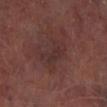The lesion was photographed on a routine skin check and not biopsied; there is no pathology result. The recorded lesion diameter is about 4.5 mm. The lesion-visualizer software estimated an automated nevus-likeness rating near 0 out of 100 and a detector confidence of about 95 out of 100 that the crop contains a lesion. Captured under cross-polarized illumination. The lesion is located on the left lower leg. A male patient, approximately 65 years of age. A 15 mm crop from a total-body photograph taken for skin-cancer surveillance.